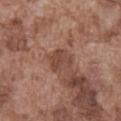<lesion>
  <biopsy_status>not biopsied; imaged during a skin examination</biopsy_status>
  <lesion_size>
    <long_diameter_mm_approx>3.0</long_diameter_mm_approx>
  </lesion_size>
  <image>
    <source>total-body photography crop</source>
    <field_of_view_mm>15</field_of_view_mm>
  </image>
  <site>abdomen</site>
  <patient>
    <sex>male</sex>
    <age_approx>75</age_approx>
  </patient>
  <lighting>white-light</lighting>
</lesion>